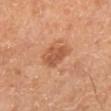workup: catalogued during a skin exam; not biopsied
body site: the right lower leg
patient: male, aged 58 to 62
acquisition: total-body-photography crop, ~15 mm field of view